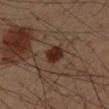No biopsy was performed on this lesion — it was imaged during a full skin examination and was not determined to be concerning. Automated tile analysis of the lesion measured a shape eccentricity near 0.45 and a symmetry-axis asymmetry near 0.2. The software also gave a mean CIELAB color near L≈24 a*≈18 b*≈23, a lesion–skin lightness drop of about 10, and a lesion-to-skin contrast of about 11.5 (normalized; higher = more distinct). The lesion's longest dimension is about 2.5 mm. The patient is a male in their 50s. A 15 mm close-up tile from a total-body photography series done for melanoma screening. Located on the right upper arm.